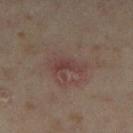Recorded during total-body skin imaging; not selected for excision or biopsy. On the left thigh. The total-body-photography lesion software estimated an area of roughly 4 mm² and a symmetry-axis asymmetry near 0.55. The software also gave a border-irregularity index near 5.5/10 and radial color variation of about 0. The analysis additionally found a nevus-likeness score of about 0/100. A roughly 15 mm field-of-view crop from a total-body skin photograph. A female patient aged 33–37.